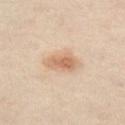follow-up — catalogued during a skin exam; not biopsied.